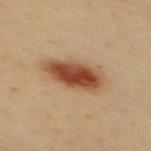<lesion>
  <biopsy_status>not biopsied; imaged during a skin examination</biopsy_status>
  <lesion_size>
    <long_diameter_mm_approx>6.0</long_diameter_mm_approx>
  </lesion_size>
  <patient>
    <sex>male</sex>
    <age_approx>40</age_approx>
  </patient>
  <site>mid back</site>
  <image>
    <source>total-body photography crop</source>
    <field_of_view_mm>15</field_of_view_mm>
  </image>
</lesion>